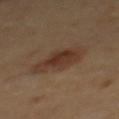follow-up = total-body-photography surveillance lesion; no biopsy
image-analysis metrics = a lesion area of about 15 mm², a shape eccentricity near 0.8, and a symmetry-axis asymmetry near 0.2; a lesion color around L≈35 a*≈17 b*≈25 in CIELAB, roughly 8 lightness units darker than nearby skin, and a normalized lesion–skin contrast near 7.5; border irregularity of about 2.5 on a 0–10 scale, a color-variation rating of about 4/10, and peripheral color asymmetry of about 1
location = the mid back
image = ~15 mm crop, total-body skin-cancer survey
patient = male, in their mid- to late 60s
lesion size = ~5.5 mm (longest diameter)